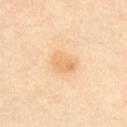Assessment: Captured during whole-body skin photography for melanoma surveillance; the lesion was not biopsied. Acquisition and patient details: About 3 mm across. The subject is a male about 50 years old. The lesion is on the front of the torso. An algorithmic analysis of the crop reported a lesion color around L≈72 a*≈19 b*≈41 in CIELAB, roughly 8 lightness units darker than nearby skin, and a normalized border contrast of about 6. The software also gave a color-variation rating of about 3/10 and radial color variation of about 1. The software also gave a classifier nevus-likeness of about 60/100. This image is a 15 mm lesion crop taken from a total-body photograph.